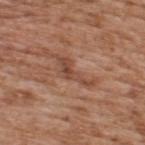notes: catalogued during a skin exam; not biopsied | body site: the back | lesion size: ~4.5 mm (longest diameter) | image: ~15 mm tile from a whole-body skin photo | tile lighting: white-light | image-analysis metrics: an average lesion color of about L≈46 a*≈22 b*≈29 (CIELAB), roughly 9 lightness units darker than nearby skin, and a normalized border contrast of about 7; a nevus-likeness score of about 0/100 | subject: male, aged 63–67.